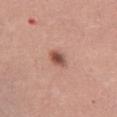follow-up: no biopsy performed (imaged during a skin exam)
image source: ~15 mm crop, total-body skin-cancer survey
automated lesion analysis: a lesion area of about 4 mm², an outline eccentricity of about 0.7 (0 = round, 1 = elongated), and a symmetry-axis asymmetry near 0.25; a mean CIELAB color near L≈52 a*≈24 b*≈27 and a normalized border contrast of about 9; border irregularity of about 2 on a 0–10 scale and a peripheral color-asymmetry measure near 1.5
diameter: ≈2.5 mm
tile lighting: white-light illumination
anatomic site: the leg
patient: female, aged 33 to 37